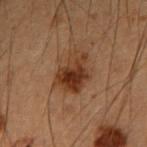Q: Is there a histopathology result?
A: total-body-photography surveillance lesion; no biopsy
Q: What is the lesion's diameter?
A: about 4.5 mm
Q: Illumination type?
A: cross-polarized illumination
Q: Lesion location?
A: the right forearm
Q: What are the patient's age and sex?
A: male, aged around 55
Q: How was this image acquired?
A: ~15 mm crop, total-body skin-cancer survey